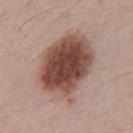Notes:
* biopsy status: imaged on a skin check; not biopsied
* subject: male, aged 38 to 42
* diameter: ≈7.5 mm
* acquisition: ~15 mm tile from a whole-body skin photo
* automated lesion analysis: a border-irregularity index near 2/10, internal color variation of about 6.5 on a 0–10 scale, and radial color variation of about 1.5; an automated nevus-likeness rating near 90 out of 100 and a lesion-detection confidence of about 100/100
* body site: the abdomen
* lighting: white-light illumination